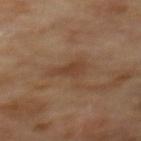Notes:
- biopsy status — imaged on a skin check; not biopsied
- site — the back
- image — ~15 mm crop, total-body skin-cancer survey
- patient — male, aged 68 to 72